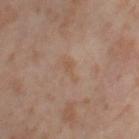Q: Was a biopsy performed?
A: no biopsy performed (imaged during a skin exam)
Q: What is the anatomic site?
A: the right thigh
Q: What lighting was used for the tile?
A: cross-polarized
Q: Patient demographics?
A: female, roughly 55 years of age
Q: What kind of image is this?
A: 15 mm crop, total-body photography
Q: What did automated image analysis measure?
A: a symmetry-axis asymmetry near 0.45; a lesion–skin lightness drop of about 5 and a normalized border contrast of about 5; border irregularity of about 5 on a 0–10 scale, a within-lesion color-variation index near 0/10, and peripheral color asymmetry of about 0